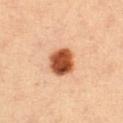No biopsy was performed on this lesion — it was imaged during a full skin examination and was not determined to be concerning. A female patient approximately 50 years of age. A 15 mm close-up extracted from a 3D total-body photography capture. The lesion is located on the leg.